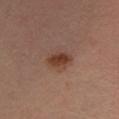Assessment: The lesion was tiled from a total-body skin photograph and was not biopsied. Acquisition and patient details: A male patient about 60 years old. The lesion is on the right lower leg. The lesion's longest dimension is about 3.5 mm. The total-body-photography lesion software estimated an area of roughly 5 mm², an outline eccentricity of about 0.8 (0 = round, 1 = elongated), and two-axis asymmetry of about 0.15. It also reported a border-irregularity index near 2/10 and a color-variation rating of about 2/10. The analysis additionally found an automated nevus-likeness rating near 100 out of 100 and a lesion-detection confidence of about 100/100. A 15 mm close-up extracted from a 3D total-body photography capture.